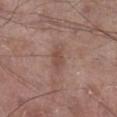Q: Is there a histopathology result?
A: no biopsy performed (imaged during a skin exam)
Q: Where on the body is the lesion?
A: the leg
Q: Patient demographics?
A: male, aged approximately 70
Q: How was this image acquired?
A: ~15 mm crop, total-body skin-cancer survey